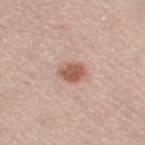biopsy_status: not biopsied; imaged during a skin examination
image:
  source: total-body photography crop
  field_of_view_mm: 15
lighting: white-light
patient:
  sex: male
  age_approx: 70
site: right thigh
lesion_size:
  long_diameter_mm_approx: 3.5